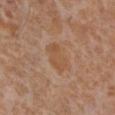{"patient": {"sex": "female", "age_approx": 55}, "automated_metrics": {"area_mm2_approx": 8.0, "eccentricity": 0.7, "shape_asymmetry": 0.2, "cielab_L": 52, "cielab_a": 20, "cielab_b": 33, "vs_skin_darker_L": 6.0, "vs_skin_contrast_norm": 5.5, "border_irregularity_0_10": 2.5, "color_variation_0_10": 2.0, "nevus_likeness_0_100": 0, "lesion_detection_confidence_0_100": 100}, "site": "left lower leg", "image": {"source": "total-body photography crop", "field_of_view_mm": 15}}